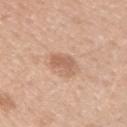Case summary:
* notes — imaged on a skin check; not biopsied
* lesion diameter — about 3.5 mm
* tile lighting — white-light illumination
* location — the right upper arm
* TBP lesion metrics — a lesion area of about 6 mm² and an eccentricity of roughly 0.65
* image — ~15 mm tile from a whole-body skin photo
* subject — female, about 40 years old